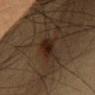Q: Is there a histopathology result?
A: imaged on a skin check; not biopsied
Q: What kind of image is this?
A: 15 mm crop, total-body photography
Q: What are the patient's age and sex?
A: male, aged 58 to 62
Q: Where on the body is the lesion?
A: the front of the torso
Q: Illumination type?
A: cross-polarized
Q: How large is the lesion?
A: about 4 mm